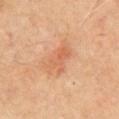Background: The subject is a male aged around 65. About 4.5 mm across. Cropped from a total-body skin-imaging series; the visible field is about 15 mm. The lesion is on the chest.